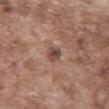Case summary:
- follow-up — no biopsy performed (imaged during a skin exam)
- image-analysis metrics — a lesion area of about 4 mm², an outline eccentricity of about 0.6 (0 = round, 1 = elongated), and a shape-asymmetry score of about 0.25 (0 = symmetric); an average lesion color of about L≈47 a*≈18 b*≈23 (CIELAB), roughly 11 lightness units darker than nearby skin, and a normalized lesion–skin contrast near 8; a border-irregularity index near 2/10, a color-variation rating of about 5/10, and peripheral color asymmetry of about 1.5; an automated nevus-likeness rating near 10 out of 100 and lesion-presence confidence of about 100/100
- illumination — white-light
- site — the abdomen
- image — 15 mm crop, total-body photography
- diameter — ~2.5 mm (longest diameter)
- patient — male, in their mid- to late 70s